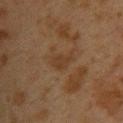Case summary:
- notes · catalogued during a skin exam; not biopsied
- anatomic site · the upper back
- subject · female, roughly 40 years of age
- image source · total-body-photography crop, ~15 mm field of view